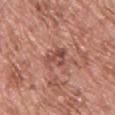Part of a total-body skin-imaging series; this lesion was reviewed on a skin check and was not flagged for biopsy. On the upper back. A male patient aged approximately 65. A 15 mm crop from a total-body photograph taken for skin-cancer surveillance. Captured under white-light illumination. Approximately 3 mm at its widest.